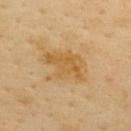Findings:
- biopsy status · total-body-photography surveillance lesion; no biopsy
- acquisition · total-body-photography crop, ~15 mm field of view
- subject · female, approximately 50 years of age
- site · the upper back